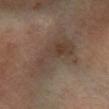Impression:
Part of a total-body skin-imaging series; this lesion was reviewed on a skin check and was not flagged for biopsy.
Context:
This image is a 15 mm lesion crop taken from a total-body photograph. A female patient approximately 70 years of age. Located on the right lower leg.